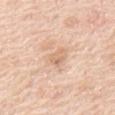No biopsy was performed on this lesion — it was imaged during a full skin examination and was not determined to be concerning. The subject is a male in their mid- to late 60s. A region of skin cropped from a whole-body photographic capture, roughly 15 mm wide. The lesion's longest dimension is about 2.5 mm. An algorithmic analysis of the crop reported border irregularity of about 2.5 on a 0–10 scale. The software also gave a nevus-likeness score of about 0/100 and lesion-presence confidence of about 100/100. On the chest.